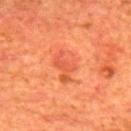Recorded during total-body skin imaging; not selected for excision or biopsy.
The subject is a male in their mid-60s.
A 15 mm crop from a total-body photograph taken for skin-cancer surveillance.
Located on the mid back.
About 4 mm across.
Captured under cross-polarized illumination.